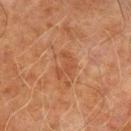This lesion was catalogued during total-body skin photography and was not selected for biopsy.
About 3.5 mm across.
The lesion is on the left upper arm.
A male patient, aged 68–72.
Imaged with cross-polarized lighting.
A 15 mm close-up tile from a total-body photography series done for melanoma screening.